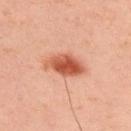No biopsy was performed on this lesion — it was imaged during a full skin examination and was not determined to be concerning.